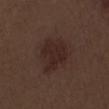Part of a total-body skin-imaging series; this lesion was reviewed on a skin check and was not flagged for biopsy. A male subject aged approximately 70. A 15 mm close-up extracted from a 3D total-body photography capture. From the right upper arm.